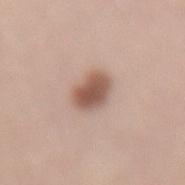– workup: imaged on a skin check; not biopsied
– size: ~3.5 mm (longest diameter)
– imaging modality: 15 mm crop, total-body photography
– site: the lower back
– patient: female, aged 43–47